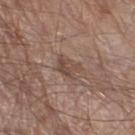Measured at roughly 3 mm in maximum diameter. The lesion is on the right thigh. The lesion-visualizer software estimated an outline eccentricity of about 0.65 (0 = round, 1 = elongated) and a symmetry-axis asymmetry near 0.45. It also reported a lesion-detection confidence of about 100/100. This is a white-light tile. A male patient, approximately 80 years of age. A 15 mm crop from a total-body photograph taken for skin-cancer surveillance.